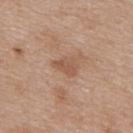Clinical impression: Imaged during a routine full-body skin examination; the lesion was not biopsied and no histopathology is available. Acquisition and patient details: Measured at roughly 2.5 mm in maximum diameter. On the upper back. A female patient, aged approximately 40. A region of skin cropped from a whole-body photographic capture, roughly 15 mm wide. The total-body-photography lesion software estimated a border-irregularity rating of about 3/10, a within-lesion color-variation index near 1.5/10, and a peripheral color-asymmetry measure near 0.5. And it measured a detector confidence of about 100 out of 100 that the crop contains a lesion.